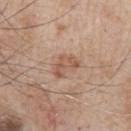• follow-up — catalogued during a skin exam; not biopsied
• subject — male, aged around 65
• image source — ~15 mm tile from a whole-body skin photo
• tile lighting — white-light illumination
• body site — the right upper arm
• lesion diameter — ~3 mm (longest diameter)